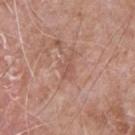biopsy status — no biopsy performed (imaged during a skin exam) | automated metrics — an area of roughly 3 mm², an eccentricity of roughly 0.85, and two-axis asymmetry of about 0.4; a lesion–skin lightness drop of about 6; border irregularity of about 4.5 on a 0–10 scale and peripheral color asymmetry of about 0; lesion-presence confidence of about 95/100 | acquisition — ~15 mm crop, total-body skin-cancer survey | patient — male, roughly 65 years of age | diameter — ~2.5 mm (longest diameter) | site — the front of the torso.